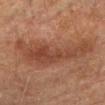<lesion>
<biopsy_status>not biopsied; imaged during a skin examination</biopsy_status>
<site>head or neck</site>
<patient>
  <sex>female</sex>
  <age_approx>80</age_approx>
</patient>
<image>
  <source>total-body photography crop</source>
  <field_of_view_mm>15</field_of_view_mm>
</image>
<automated_metrics>
  <area_mm2_approx>31.0</area_mm2_approx>
  <eccentricity>0.95</eccentricity>
  <shape_asymmetry>0.45</shape_asymmetry>
  <cielab_L>41</cielab_L>
  <cielab_a>21</cielab_a>
  <cielab_b>29</cielab_b>
  <vs_skin_darker_L>8.0</vs_skin_darker_L>
  <border_irregularity_0_10>7.0</border_irregularity_0_10>
  <color_variation_0_10>4.0</color_variation_0_10>
  <peripheral_color_asymmetry>1.0</peripheral_color_asymmetry>
  <nevus_likeness_0_100>15</nevus_likeness_0_100>
  <lesion_detection_confidence_0_100>100</lesion_detection_confidence_0_100>
</automated_metrics>
</lesion>